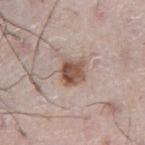• biopsy status: imaged on a skin check; not biopsied
• patient: male, aged approximately 75
• acquisition: total-body-photography crop, ~15 mm field of view
• illumination: white-light illumination
• automated lesion analysis: a footprint of about 7.5 mm² and a shape-asymmetry score of about 0.3 (0 = symmetric); roughly 14 lightness units darker than nearby skin and a lesion-to-skin contrast of about 10 (normalized; higher = more distinct); a classifier nevus-likeness of about 95/100 and a lesion-detection confidence of about 100/100
• location: the abdomen